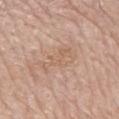| field | value |
|---|---|
| workup | total-body-photography surveillance lesion; no biopsy |